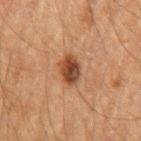Part of a total-body skin-imaging series; this lesion was reviewed on a skin check and was not flagged for biopsy. On the left upper arm. A male patient aged 63–67. This image is a 15 mm lesion crop taken from a total-body photograph.